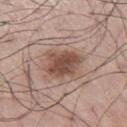An algorithmic analysis of the crop reported a border-irregularity index near 2.5/10 and peripheral color asymmetry of about 1.5. On the lower back. A male subject, about 70 years old. The tile uses white-light illumination. A lesion tile, about 15 mm wide, cut from a 3D total-body photograph.